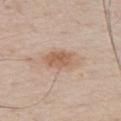{
  "biopsy_status": "not biopsied; imaged during a skin examination",
  "lighting": "white-light",
  "patient": {
    "sex": "male",
    "age_approx": 55
  },
  "site": "chest",
  "image": {
    "source": "total-body photography crop",
    "field_of_view_mm": 15
  },
  "lesion_size": {
    "long_diameter_mm_approx": 4.5
  }
}